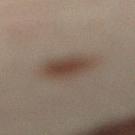Assessment:
The lesion was photographed on a routine skin check and not biopsied; there is no pathology result.
Context:
On the left leg. This is a cross-polarized tile. A close-up tile cropped from a whole-body skin photograph, about 15 mm across. Automated image analysis of the tile measured an eccentricity of roughly 0.65. And it measured an automated nevus-likeness rating near 100 out of 100 and a detector confidence of about 100 out of 100 that the crop contains a lesion. The patient is a male aged 48–52.